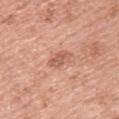biopsy status: imaged on a skin check; not biopsied
imaging modality: ~15 mm crop, total-body skin-cancer survey
lesion size: about 3.5 mm
lighting: white-light
patient: female, roughly 50 years of age
automated metrics: an eccentricity of roughly 0.9 and a symmetry-axis asymmetry near 0.2; a mean CIELAB color near L≈59 a*≈24 b*≈30, about 10 CIELAB-L* units darker than the surrounding skin, and a normalized lesion–skin contrast near 6.5; a color-variation rating of about 2/10 and a peripheral color-asymmetry measure near 1
anatomic site: the left upper arm